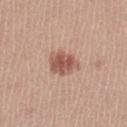Recorded during total-body skin imaging; not selected for excision or biopsy. From the leg. A 15 mm crop from a total-body photograph taken for skin-cancer surveillance. The total-body-photography lesion software estimated a footprint of about 8.5 mm² and a shape-asymmetry score of about 0.25 (0 = symmetric). It also reported a mean CIELAB color near L≈55 a*≈23 b*≈27, about 12 CIELAB-L* units darker than the surrounding skin, and a normalized lesion–skin contrast near 8. The recorded lesion diameter is about 3.5 mm. The patient is a female approximately 20 years of age.